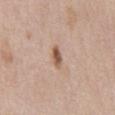* notes: no biopsy performed (imaged during a skin exam)
* lighting: white-light illumination
* body site: the chest
* image: 15 mm crop, total-body photography
* TBP lesion metrics: a footprint of about 3.5 mm², a shape eccentricity near 0.85, and a shape-asymmetry score of about 0.3 (0 = symmetric); a mean CIELAB color near L≈56 a*≈19 b*≈29, roughly 13 lightness units darker than nearby skin, and a lesion-to-skin contrast of about 9 (normalized; higher = more distinct); a border-irregularity index near 3/10 and a peripheral color-asymmetry measure near 1.5
* lesion size: ~3 mm (longest diameter)
* subject: male, approximately 60 years of age